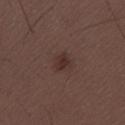A lesion tile, about 15 mm wide, cut from a 3D total-body photograph. Measured at roughly 2.5 mm in maximum diameter. The lesion is located on the lower back. A male patient roughly 30 years of age. Imaged with white-light lighting. Automated tile analysis of the lesion measured a lesion–skin lightness drop of about 7 and a lesion-to-skin contrast of about 7 (normalized; higher = more distinct).